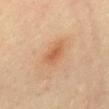This lesion was catalogued during total-body skin photography and was not selected for biopsy. Cropped from a whole-body photographic skin survey; the tile spans about 15 mm. Approximately 2.5 mm at its widest. This is a cross-polarized tile. Automated image analysis of the tile measured a lesion area of about 3.5 mm², a shape eccentricity near 0.8, and a symmetry-axis asymmetry near 0.3. On the mid back. A female patient, about 40 years old.